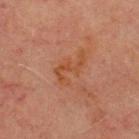follow-up=catalogued during a skin exam; not biopsied
tile lighting=cross-polarized
image source=total-body-photography crop, ~15 mm field of view
image-analysis metrics=a lesion area of about 5.5 mm², a shape eccentricity near 0.9, and two-axis asymmetry of about 0.7; a color-variation rating of about 0/10 and radial color variation of about 0
location=the chest
subject=male, roughly 70 years of age